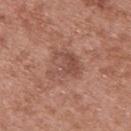{"biopsy_status": "not biopsied; imaged during a skin examination", "lesion_size": {"long_diameter_mm_approx": 4.0}, "lighting": "white-light", "patient": {"sex": "female", "age_approx": 40}, "automated_metrics": {"area_mm2_approx": 9.5, "eccentricity": 0.45, "shape_asymmetry": 0.45}, "image": {"source": "total-body photography crop", "field_of_view_mm": 15}, "site": "upper back"}